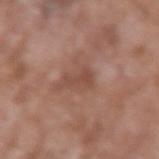notes: imaged on a skin check; not biopsied | lesion size: ≈3 mm | image: ~15 mm crop, total-body skin-cancer survey | tile lighting: white-light illumination | automated metrics: a footprint of about 4 mm², a shape eccentricity near 0.75, and a symmetry-axis asymmetry near 0.55; a border-irregularity index near 5.5/10, internal color variation of about 1.5 on a 0–10 scale, and radial color variation of about 0.5 | patient: male, aged approximately 60 | location: the left upper arm.